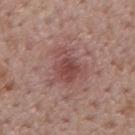<record>
  <biopsy_status>not biopsied; imaged during a skin examination</biopsy_status>
  <image>
    <source>total-body photography crop</source>
    <field_of_view_mm>15</field_of_view_mm>
  </image>
  <lighting>white-light</lighting>
  <patient>
    <sex>male</sex>
    <age_approx>55</age_approx>
  </patient>
  <site>mid back</site>
</record>